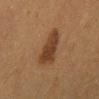follow-up = no biopsy performed (imaged during a skin exam) | location = the back | tile lighting = cross-polarized | subject = female, aged approximately 50 | image = ~15 mm crop, total-body skin-cancer survey | lesion diameter = ~5 mm (longest diameter).